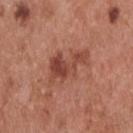{
  "biopsy_status": "not biopsied; imaged during a skin examination",
  "site": "back",
  "patient": {
    "sex": "male",
    "age_approx": 55
  },
  "lesion_size": {
    "long_diameter_mm_approx": 5.5
  },
  "image": {
    "source": "total-body photography crop",
    "field_of_view_mm": 15
  }
}